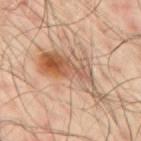Recorded during total-body skin imaging; not selected for excision or biopsy. Automated tile analysis of the lesion measured roughly 12 lightness units darker than nearby skin. The analysis additionally found a border-irregularity rating of about 8.5/10, internal color variation of about 9.5 on a 0–10 scale, and radial color variation of about 3. The software also gave an automated nevus-likeness rating near 100 out of 100. Longest diameter approximately 9.5 mm. From the mid back. The subject is a male about 50 years old. A close-up tile cropped from a whole-body skin photograph, about 15 mm across. The tile uses cross-polarized illumination.